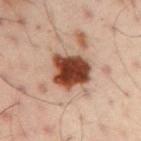Q: Is there a histopathology result?
A: total-body-photography surveillance lesion; no biopsy
Q: What is the imaging modality?
A: total-body-photography crop, ~15 mm field of view
Q: Who is the patient?
A: male, in their 50s
Q: Automated lesion metrics?
A: a lesion area of about 15 mm², a shape eccentricity near 0.45, and a symmetry-axis asymmetry near 0.2; a border-irregularity index near 2/10, a color-variation rating of about 6/10, and peripheral color asymmetry of about 1.5
Q: Where on the body is the lesion?
A: the left arm
Q: Lesion size?
A: about 4.5 mm
Q: Illumination type?
A: cross-polarized